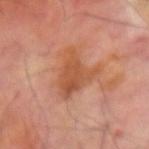Impression:
Captured during whole-body skin photography for melanoma surveillance; the lesion was not biopsied.
Image and clinical context:
This image is a 15 mm lesion crop taken from a total-body photograph. A male patient aged approximately 70. This is a cross-polarized tile. An algorithmic analysis of the crop reported a lesion-detection confidence of about 100/100. The lesion is on the right forearm. Approximately 5 mm at its widest.